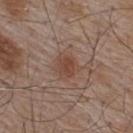{"lighting": "white-light", "lesion_size": {"long_diameter_mm_approx": 3.0}, "site": "upper back", "image": {"source": "total-body photography crop", "field_of_view_mm": 15}, "patient": {"sex": "male", "age_approx": 55}}